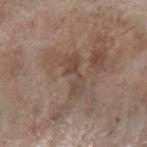Q: Was a biopsy performed?
A: no biopsy performed (imaged during a skin exam)
Q: What are the patient's age and sex?
A: female, aged around 75
Q: What is the imaging modality?
A: ~15 mm tile from a whole-body skin photo
Q: Lesion location?
A: the left forearm
Q: What is the lesion's diameter?
A: about 4.5 mm
Q: Automated lesion metrics?
A: a border-irregularity index near 6.5/10 and a color-variation rating of about 3/10; a classifier nevus-likeness of about 0/100 and a lesion-detection confidence of about 95/100